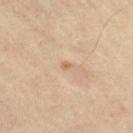notes — catalogued during a skin exam; not biopsied | image-analysis metrics — a lesion area of about 1 mm² and a shape eccentricity near 0.8; a border-irregularity index near 3/10 and a peripheral color-asymmetry measure near 0; a classifier nevus-likeness of about 0/100 and lesion-presence confidence of about 100/100 | anatomic site — the leg | tile lighting — cross-polarized illumination | subject — female, in their mid-60s | lesion diameter — ~1 mm (longest diameter) | image source — ~15 mm tile from a whole-body skin photo.